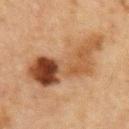<case>
<biopsy_status>not biopsied; imaged during a skin examination</biopsy_status>
<lesion_size>
  <long_diameter_mm_approx>10.0</long_diameter_mm_approx>
</lesion_size>
<image>
  <source>total-body photography crop</source>
  <field_of_view_mm>15</field_of_view_mm>
</image>
<patient>
  <sex>male</sex>
  <age_approx>65</age_approx>
</patient>
<lighting>cross-polarized</lighting>
<site>front of the torso</site>
</case>